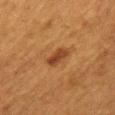The lesion was photographed on a routine skin check and not biopsied; there is no pathology result. A 15 mm close-up tile from a total-body photography series done for melanoma screening. A female patient aged approximately 40. Captured under cross-polarized illumination. The lesion is located on the chest. About 3 mm across. Automated image analysis of the tile measured a footprint of about 4.5 mm², an outline eccentricity of about 0.85 (0 = round, 1 = elongated), and a shape-asymmetry score of about 0.2 (0 = symmetric). And it measured an average lesion color of about L≈35 a*≈21 b*≈32 (CIELAB), about 9 CIELAB-L* units darker than the surrounding skin, and a lesion-to-skin contrast of about 8.5 (normalized; higher = more distinct). The software also gave a border-irregularity rating of about 2/10, internal color variation of about 3 on a 0–10 scale, and radial color variation of about 1.